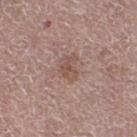No biopsy was performed on this lesion — it was imaged during a full skin examination and was not determined to be concerning. A roughly 15 mm field-of-view crop from a total-body skin photograph. Located on the right thigh. About 2.5 mm across. The total-body-photography lesion software estimated a footprint of about 4 mm², an eccentricity of roughly 0.75, and two-axis asymmetry of about 0.45. The software also gave a lesion color around L≈51 a*≈18 b*≈25 in CIELAB, about 8 CIELAB-L* units darker than the surrounding skin, and a lesion-to-skin contrast of about 6 (normalized; higher = more distinct). A female subject, in their mid- to late 60s. Imaged with white-light lighting.